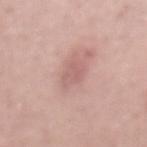follow-up — catalogued during a skin exam; not biopsied | image-analysis metrics — an area of roughly 5 mm², an outline eccentricity of about 0.6 (0 = round, 1 = elongated), and a shape-asymmetry score of about 0.3 (0 = symmetric); an average lesion color of about L≈60 a*≈22 b*≈23 (CIELAB), roughly 7 lightness units darker than nearby skin, and a normalized border contrast of about 5; a classifier nevus-likeness of about 0/100 | tile lighting — white-light | imaging modality — ~15 mm tile from a whole-body skin photo | subject — male, aged approximately 40 | lesion size — ~3 mm (longest diameter) | location — the mid back.